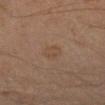Clinical impression:
The lesion was photographed on a routine skin check and not biopsied; there is no pathology result.
Context:
Cropped from a whole-body photographic skin survey; the tile spans about 15 mm. About 2.5 mm across. Imaged with cross-polarized lighting. A male subject, approximately 45 years of age. The lesion is on the left upper arm.